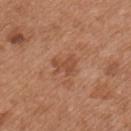Case summary:
• follow-up · imaged on a skin check; not biopsied
• patient · male, about 55 years old
• body site · the chest
• image source · 15 mm crop, total-body photography
• tile lighting · white-light illumination
• lesion size · ~3 mm (longest diameter)